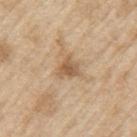This lesion was catalogued during total-body skin photography and was not selected for biopsy. This image is a 15 mm lesion crop taken from a total-body photograph. Automated tile analysis of the lesion measured a shape eccentricity near 0.3. Located on the left upper arm. A male patient, roughly 70 years of age. The recorded lesion diameter is about 2.5 mm.